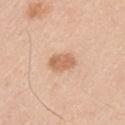Recorded during total-body skin imaging; not selected for excision or biopsy.
The lesion is located on the left upper arm.
A region of skin cropped from a whole-body photographic capture, roughly 15 mm wide.
A male patient, aged around 35.